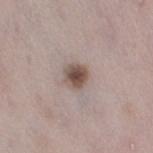The lesion was tiled from a total-body skin photograph and was not biopsied. The lesion is on the leg. A female subject in their 30s. A region of skin cropped from a whole-body photographic capture, roughly 15 mm wide.